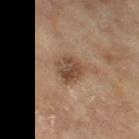Clinical impression:
Captured during whole-body skin photography for melanoma surveillance; the lesion was not biopsied.
Context:
Located on the left thigh. Cropped from a whole-body photographic skin survey; the tile spans about 15 mm. The tile uses cross-polarized illumination. A female subject, in their mid- to late 70s.